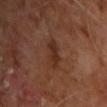Part of a total-body skin-imaging series; this lesion was reviewed on a skin check and was not flagged for biopsy.
Automated tile analysis of the lesion measured a lesion area of about 5 mm², a shape eccentricity near 0.8, and a shape-asymmetry score of about 0.25 (0 = symmetric). The software also gave a lesion color around L≈28 a*≈20 b*≈26 in CIELAB and a lesion–skin lightness drop of about 7. The analysis additionally found lesion-presence confidence of about 100/100.
About 3 mm across.
On the upper back.
The subject is a male aged 58–62.
Imaged with cross-polarized lighting.
Cropped from a whole-body photographic skin survey; the tile spans about 15 mm.